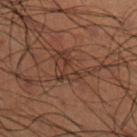Acquisition and patient details: Cropped from a total-body skin-imaging series; the visible field is about 15 mm. A male subject, in their 50s. The tile uses cross-polarized illumination. Automated image analysis of the tile measured an area of roughly 9 mm², a shape eccentricity near 0.65, and two-axis asymmetry of about 0.6. And it measured a lesion color around L≈27 a*≈15 b*≈21 in CIELAB, roughly 5 lightness units darker than nearby skin, and a lesion-to-skin contrast of about 5.5 (normalized; higher = more distinct). The analysis additionally found an automated nevus-likeness rating near 0 out of 100 and lesion-presence confidence of about 55/100. Measured at roughly 4.5 mm in maximum diameter. The lesion is located on the right lower leg.